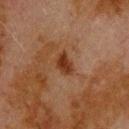  biopsy_status: not biopsied; imaged during a skin examination
  patient:
    sex: male
    age_approx: 80
  site: upper back
  image:
    source: total-body photography crop
    field_of_view_mm: 15